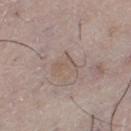Q: Is there a histopathology result?
A: imaged on a skin check; not biopsied
Q: Patient demographics?
A: male, in their mid- to late 50s
Q: How was this image acquired?
A: ~15 mm tile from a whole-body skin photo
Q: What lighting was used for the tile?
A: white-light
Q: Where on the body is the lesion?
A: the left thigh